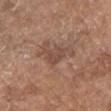Part of a total-body skin-imaging series; this lesion was reviewed on a skin check and was not flagged for biopsy. Captured under white-light illumination. The subject is a male about 80 years old. On the head or neck. A 15 mm close-up tile from a total-body photography series done for melanoma screening.